The patient is a female roughly 50 years of age · the lesion is on the left thigh · a 15 mm crop from a total-body photograph taken for skin-cancer surveillance:
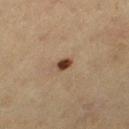lighting: cross-polarized
diagnosis:
  histopathology: dysplastic (Clark) nevus
  malignancy: benign
  taxonomic_path:
    - Benign
    - Benign melanocytic proliferations
    - Nevus
    - Nevus, Atypical, Dysplastic, or Clark